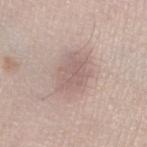Assessment:
Captured during whole-body skin photography for melanoma surveillance; the lesion was not biopsied.
Clinical summary:
A female patient aged around 60. Located on the leg. Longest diameter approximately 4 mm. Cropped from a total-body skin-imaging series; the visible field is about 15 mm.